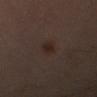Context:
Approximately 3 mm at its widest. This image is a 15 mm lesion crop taken from a total-body photograph. A male patient, about 50 years old. The lesion is on the left upper arm.